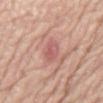The lesion was photographed on a routine skin check and not biopsied; there is no pathology result. Located on the abdomen. Cropped from a total-body skin-imaging series; the visible field is about 15 mm. A female patient aged 68 to 72. Captured under white-light illumination. Measured at roughly 3 mm in maximum diameter.